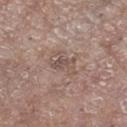{
  "biopsy_status": "not biopsied; imaged during a skin examination",
  "site": "right lower leg",
  "image": {
    "source": "total-body photography crop",
    "field_of_view_mm": 15
  },
  "automated_metrics": {
    "area_mm2_approx": 5.5,
    "eccentricity": 0.7,
    "shape_asymmetry": 0.3,
    "cielab_L": 51,
    "cielab_a": 15,
    "cielab_b": 22,
    "vs_skin_darker_L": 7.0,
    "vs_skin_contrast_norm": 5.5,
    "border_irregularity_0_10": 4.0,
    "color_variation_0_10": 3.5,
    "peripheral_color_asymmetry": 1.5
  },
  "lighting": "white-light",
  "patient": {
    "sex": "female",
    "age_approx": 75
  }
}